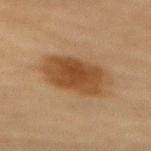Notes:
• workup · imaged on a skin check; not biopsied
• subject · female, approximately 80 years of age
• tile lighting · cross-polarized illumination
• size · ≈6.5 mm
• location · the mid back
• image · ~15 mm tile from a whole-body skin photo